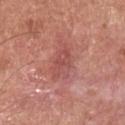biopsy_status: not biopsied; imaged during a skin examination
automated_metrics:
  area_mm2_approx: 8.0
  shape_asymmetry: 0.35
  border_irregularity_0_10: 4.0
  color_variation_0_10: 2.5
  peripheral_color_asymmetry: 0.5
  nevus_likeness_0_100: 0
  lesion_detection_confidence_0_100: 95
patient:
  sex: male
  age_approx: 55
lighting: white-light
image:
  source: total-body photography crop
  field_of_view_mm: 15
site: front of the torso
lesion_size:
  long_diameter_mm_approx: 4.5